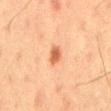Recorded during total-body skin imaging; not selected for excision or biopsy. The subject is a male aged 58 to 62. A lesion tile, about 15 mm wide, cut from a 3D total-body photograph. The lesion is on the mid back. Approximately 2.5 mm at its widest. Automated image analysis of the tile measured about 10 CIELAB-L* units darker than the surrounding skin and a normalized lesion–skin contrast near 8. The analysis additionally found lesion-presence confidence of about 100/100. This is a cross-polarized tile.